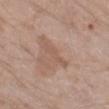Case summary:
- workup: imaged on a skin check; not biopsied
- anatomic site: the left thigh
- subject: male, aged approximately 65
- diameter: about 4.5 mm
- image source: total-body-photography crop, ~15 mm field of view
- tile lighting: white-light